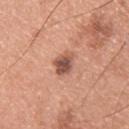Case summary:
• notes: no biopsy performed (imaged during a skin exam)
• body site: the upper back
• lighting: white-light
• acquisition: ~15 mm tile from a whole-body skin photo
• subject: male, aged around 30